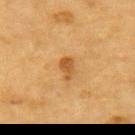Part of a total-body skin-imaging series; this lesion was reviewed on a skin check and was not flagged for biopsy. Located on the upper back. A 15 mm close-up tile from a total-body photography series done for melanoma screening. A male patient, in their mid-80s or older. Captured under cross-polarized illumination. The total-body-photography lesion software estimated an average lesion color of about L≈48 a*≈20 b*≈40 (CIELAB), a lesion–skin lightness drop of about 9, and a normalized border contrast of about 7. And it measured a border-irregularity rating of about 3/10, a color-variation rating of about 1.5/10, and a peripheral color-asymmetry measure near 0.5. The analysis additionally found a classifier nevus-likeness of about 60/100. Measured at roughly 2.5 mm in maximum diameter.